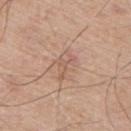This lesion was catalogued during total-body skin photography and was not selected for biopsy. A male subject, aged around 55. Captured under white-light illumination. About 4 mm across. The lesion is on the upper back. A region of skin cropped from a whole-body photographic capture, roughly 15 mm wide.